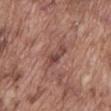workup: catalogued during a skin exam; not biopsied
patient: male, aged around 75
image-analysis metrics: a footprint of about 7.5 mm² and an outline eccentricity of about 0.9 (0 = round, 1 = elongated); a nevus-likeness score of about 0/100
body site: the front of the torso
diameter: ≈5 mm
image source: ~15 mm tile from a whole-body skin photo
tile lighting: white-light illumination